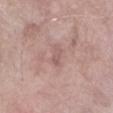<case>
  <biopsy_status>not biopsied; imaged during a skin examination</biopsy_status>
  <site>arm</site>
  <automated_metrics>
    <area_mm2_approx>3.5</area_mm2_approx>
    <eccentricity>0.85</eccentricity>
    <shape_asymmetry>0.35</shape_asymmetry>
    <nevus_likeness_0_100>0</nevus_likeness_0_100>
    <lesion_detection_confidence_0_100>95</lesion_detection_confidence_0_100>
  </automated_metrics>
  <patient>
    <sex>male</sex>
    <age_approx>60</age_approx>
  </patient>
  <image>
    <source>total-body photography crop</source>
    <field_of_view_mm>15</field_of_view_mm>
  </image>
  <lighting>white-light</lighting>
</case>